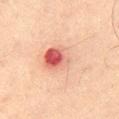Clinical impression: Imaged during a routine full-body skin examination; the lesion was not biopsied and no histopathology is available. Background: A lesion tile, about 15 mm wide, cut from a 3D total-body photograph. Captured under cross-polarized illumination. A male patient, aged 63 to 67. Automated tile analysis of the lesion measured an area of roughly 23 mm² and an eccentricity of roughly 0.65. It also reported an average lesion color of about L≈57 a*≈20 b*≈27 (CIELAB), a lesion–skin lightness drop of about 8, and a normalized border contrast of about 5.5. On the right thigh. Approximately 6.5 mm at its widest.